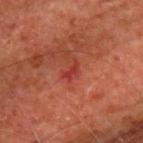biopsy status: total-body-photography surveillance lesion; no biopsy
body site: the upper back
acquisition: ~15 mm tile from a whole-body skin photo
subject: male, in their mid-60s